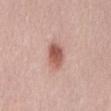Assessment:
Imaged during a routine full-body skin examination; the lesion was not biopsied and no histopathology is available.
Clinical summary:
The tile uses white-light illumination. A female patient, aged 38–42. Cropped from a total-body skin-imaging series; the visible field is about 15 mm. About 3 mm across. On the back.